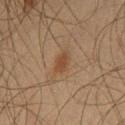No biopsy was performed on this lesion — it was imaged during a full skin examination and was not determined to be concerning. A 15 mm close-up tile from a total-body photography series done for melanoma screening. Approximately 2.5 mm at its widest. The lesion is located on the left thigh. The subject is a male approximately 45 years of age. Imaged with cross-polarized lighting.